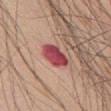Q: What are the patient's age and sex?
A: male, aged 58–62
Q: What lighting was used for the tile?
A: white-light
Q: What is the anatomic site?
A: the abdomen
Q: Lesion size?
A: ≈3.5 mm
Q: What kind of image is this?
A: 15 mm crop, total-body photography
Q: Automated lesion metrics?
A: an area of roughly 8 mm², an outline eccentricity of about 0.7 (0 = round, 1 = elongated), and two-axis asymmetry of about 0.15; an average lesion color of about L≈47 a*≈36 b*≈22 (CIELAB) and a normalized lesion–skin contrast near 11.5; a within-lesion color-variation index near 4/10; lesion-presence confidence of about 100/100